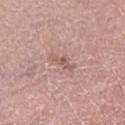The lesion was tiled from a total-body skin photograph and was not biopsied.